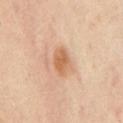Case summary:
• workup — catalogued during a skin exam; not biopsied
• site — the chest
• image source — total-body-photography crop, ~15 mm field of view
• patient — aged approximately 55
• illumination — cross-polarized illumination
• lesion size — about 3 mm
• TBP lesion metrics — a lesion area of about 6 mm², a shape eccentricity near 0.7, and a symmetry-axis asymmetry near 0.2; a border-irregularity index near 2/10, a color-variation rating of about 3/10, and radial color variation of about 1; a classifier nevus-likeness of about 75/100 and a lesion-detection confidence of about 100/100